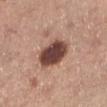| field | value |
|---|---|
| diameter | ~4.5 mm (longest diameter) |
| anatomic site | the left lower leg |
| automated metrics | a lesion area of about 13 mm², an outline eccentricity of about 0.55 (0 = round, 1 = elongated), and two-axis asymmetry of about 0.2; a border-irregularity index near 2/10 and peripheral color asymmetry of about 1 |
| image | ~15 mm tile from a whole-body skin photo |
| patient | female, about 65 years old |
| illumination | white-light |